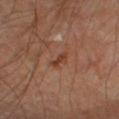No biopsy was performed on this lesion — it was imaged during a full skin examination and was not determined to be concerning.
A male subject, aged 63–67.
Captured under cross-polarized illumination.
Approximately 2.5 mm at its widest.
Cropped from a whole-body photographic skin survey; the tile spans about 15 mm.
The lesion is located on the left lower leg.